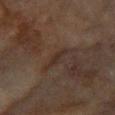The total-body-photography lesion software estimated a footprint of about 3.5 mm² and a shape eccentricity near 0.9. The analysis additionally found a mean CIELAB color near L≈24 a*≈13 b*≈19, a lesion–skin lightness drop of about 5, and a lesion-to-skin contrast of about 6 (normalized; higher = more distinct). And it measured a nevus-likeness score of about 0/100 and a lesion-detection confidence of about 65/100. A roughly 15 mm field-of-view crop from a total-body skin photograph. Imaged with cross-polarized lighting. A female subject aged approximately 80. The lesion is located on the right forearm.